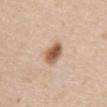Q: Is there a histopathology result?
A: catalogued during a skin exam; not biopsied
Q: Lesion size?
A: about 3 mm
Q: What lighting was used for the tile?
A: white-light illumination
Q: What are the patient's age and sex?
A: female, in their 70s
Q: What is the imaging modality?
A: total-body-photography crop, ~15 mm field of view
Q: What is the anatomic site?
A: the chest